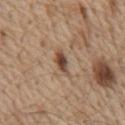Notes:
* notes — no biopsy performed (imaged during a skin exam)
* subject — male, aged 68 to 72
* lighting — white-light illumination
* site — the chest
* automated lesion analysis — a footprint of about 3.5 mm², an outline eccentricity of about 0.9 (0 = round, 1 = elongated), and a symmetry-axis asymmetry near 0.25; a mean CIELAB color near L≈46 a*≈18 b*≈29 and a normalized border contrast of about 10.5
* acquisition — 15 mm crop, total-body photography
* lesion diameter — ≈3.5 mm